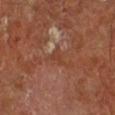  biopsy_status: not biopsied; imaged during a skin examination
  image:
    source: total-body photography crop
    field_of_view_mm: 15
  patient:
    sex: male
    age_approx: 65
  site: right lower leg